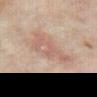Impression:
The lesion was tiled from a total-body skin photograph and was not biopsied.
Acquisition and patient details:
The lesion is on the arm. About 6 mm across. The patient is a female aged around 35. The tile uses cross-polarized illumination. The total-body-photography lesion software estimated a nevus-likeness score of about 0/100 and lesion-presence confidence of about 100/100. A roughly 15 mm field-of-view crop from a total-body skin photograph.